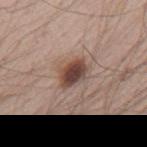biopsy status — total-body-photography surveillance lesion; no biopsy | body site — the mid back | image source — ~15 mm tile from a whole-body skin photo | patient — male, in their mid-60s.